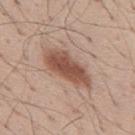Case summary:
- workup — catalogued during a skin exam; not biopsied